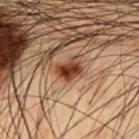location = the abdomen | subject = male, aged 53–57 | image = ~15 mm crop, total-body skin-cancer survey | size = ~3 mm (longest diameter).